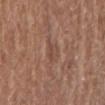The lesion was tiled from a total-body skin photograph and was not biopsied. A roughly 15 mm field-of-view crop from a total-body skin photograph. The patient is a female aged around 80. On the right upper arm.